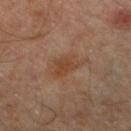Imaged during a routine full-body skin examination; the lesion was not biopsied and no histopathology is available. A 15 mm close-up extracted from a 3D total-body photography capture. About 4.5 mm across. A male subject about 55 years old. The lesion is located on the left lower leg. Automated tile analysis of the lesion measured a lesion color around L≈42 a*≈19 b*≈30 in CIELAB and about 6 CIELAB-L* units darker than the surrounding skin. The software also gave a detector confidence of about 100 out of 100 that the crop contains a lesion. Captured under cross-polarized illumination.